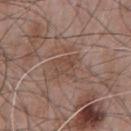Impression:
This lesion was catalogued during total-body skin photography and was not selected for biopsy.
Background:
Located on the chest. A male patient, in their mid- to late 60s. This image is a 15 mm lesion crop taken from a total-body photograph.